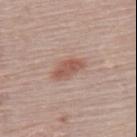Image and clinical context:
Located on the upper back. A 15 mm crop from a total-body photograph taken for skin-cancer surveillance. About 4 mm across. The subject is a female aged 63 to 67. Imaged with white-light lighting.